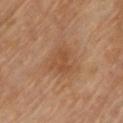<case>
  <biopsy_status>not biopsied; imaged during a skin examination</biopsy_status>
  <site>front of the torso</site>
  <lesion_size>
    <long_diameter_mm_approx>3.0</long_diameter_mm_approx>
  </lesion_size>
  <lighting>cross-polarized</lighting>
  <image>
    <source>total-body photography crop</source>
    <field_of_view_mm>15</field_of_view_mm>
  </image>
</case>